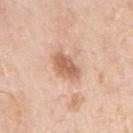Acquisition and patient details:
Longest diameter approximately 4.5 mm. A close-up tile cropped from a whole-body skin photograph, about 15 mm across. Located on the left upper arm. A male subject, about 55 years old.